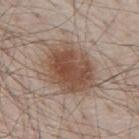notes: imaged on a skin check; not biopsied | location: the mid back | illumination: white-light illumination | TBP lesion metrics: border irregularity of about 2.5 on a 0–10 scale, a within-lesion color-variation index near 5.5/10, and peripheral color asymmetry of about 1.5; an automated nevus-likeness rating near 95 out of 100 and a detector confidence of about 100 out of 100 that the crop contains a lesion | patient: male, about 80 years old | image source: ~15 mm tile from a whole-body skin photo | diameter: ~7.5 mm (longest diameter).